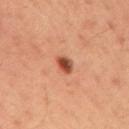workup: no biopsy performed (imaged during a skin exam); patient: male, approximately 35 years of age; image source: ~15 mm crop, total-body skin-cancer survey; location: the right upper arm.